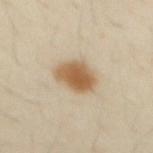Q: Is there a histopathology result?
A: no biopsy performed (imaged during a skin exam)
Q: Automated lesion metrics?
A: a border-irregularity index near 1.5/10 and a peripheral color-asymmetry measure near 1; a nevus-likeness score of about 100/100 and a lesion-detection confidence of about 100/100
Q: Where on the body is the lesion?
A: the front of the torso
Q: How was the tile lit?
A: cross-polarized illumination
Q: What are the patient's age and sex?
A: male, aged approximately 30
Q: How was this image acquired?
A: ~15 mm crop, total-body skin-cancer survey